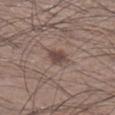Assessment: No biopsy was performed on this lesion — it was imaged during a full skin examination and was not determined to be concerning. Background: Cropped from a total-body skin-imaging series; the visible field is about 15 mm. The tile uses white-light illumination. A male patient, about 35 years old. From the right lower leg. The lesion-visualizer software estimated an average lesion color of about L≈45 a*≈16 b*≈21 (CIELAB), about 10 CIELAB-L* units darker than the surrounding skin, and a normalized border contrast of about 8. The recorded lesion diameter is about 2.5 mm.